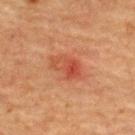Clinical impression: The lesion was tiled from a total-body skin photograph and was not biopsied. Image and clinical context: The tile uses cross-polarized illumination. Approximately 3.5 mm at its widest. The lesion-visualizer software estimated a border-irregularity rating of about 3/10, a within-lesion color-variation index near 8/10, and a peripheral color-asymmetry measure near 3. A lesion tile, about 15 mm wide, cut from a 3D total-body photograph. A female patient in their 70s. From the upper back.